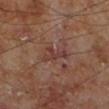image-analysis metrics: a lesion area of about 4.5 mm², an outline eccentricity of about 0.9 (0 = round, 1 = elongated), and two-axis asymmetry of about 0.5; a lesion–skin lightness drop of about 7 and a lesion-to-skin contrast of about 6 (normalized; higher = more distinct); border irregularity of about 7 on a 0–10 scale and peripheral color asymmetry of about 0; a detector confidence of about 100 out of 100 that the crop contains a lesion
patient: male, aged 68 to 72
diameter: about 4 mm
location: the leg
image: ~15 mm crop, total-body skin-cancer survey
illumination: cross-polarized